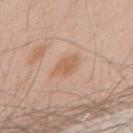Q: Is there a histopathology result?
A: total-body-photography surveillance lesion; no biopsy
Q: What is the anatomic site?
A: the upper back
Q: What did automated image analysis measure?
A: a lesion–skin lightness drop of about 8 and a lesion-to-skin contrast of about 6 (normalized; higher = more distinct); a border-irregularity index near 2.5/10 and a peripheral color-asymmetry measure near 0.5
Q: What are the patient's age and sex?
A: male, aged 68–72
Q: What kind of image is this?
A: total-body-photography crop, ~15 mm field of view